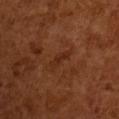  biopsy_status: not biopsied; imaged during a skin examination
  patient:
    sex: male
    age_approx: 65
  image:
    source: total-body photography crop
    field_of_view_mm: 15